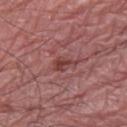The lesion is on the leg. A 15 mm close-up extracted from a 3D total-body photography capture. Captured under white-light illumination. A male subject aged 73–77.